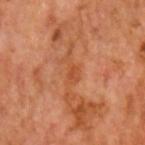Part of a total-body skin-imaging series; this lesion was reviewed on a skin check and was not flagged for biopsy. A 15 mm close-up tile from a total-body photography series done for melanoma screening. The subject is a male aged 58–62. The lesion is located on the head or neck.